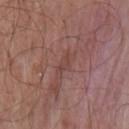{"patient": {"sex": "male", "age_approx": 55}, "site": "chest", "image": {"source": "total-body photography crop", "field_of_view_mm": 15}}